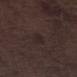Part of a total-body skin-imaging series; this lesion was reviewed on a skin check and was not flagged for biopsy. This is a white-light tile. The patient is a male aged around 70. A roughly 15 mm field-of-view crop from a total-body skin photograph. Located on the leg. Longest diameter approximately 2.5 mm.